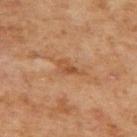No biopsy was performed on this lesion — it was imaged during a full skin examination and was not determined to be concerning.